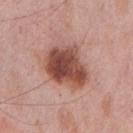Part of a total-body skin-imaging series; this lesion was reviewed on a skin check and was not flagged for biopsy.
Captured under white-light illumination.
A male subject in their mid- to late 70s.
The recorded lesion diameter is about 5.5 mm.
On the chest.
A 15 mm close-up extracted from a 3D total-body photography capture.